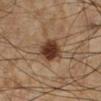Clinical impression:
Imaged during a routine full-body skin examination; the lesion was not biopsied and no histopathology is available.
Background:
Cropped from a total-body skin-imaging series; the visible field is about 15 mm. Captured under cross-polarized illumination. A male subject, roughly 60 years of age. The lesion's longest dimension is about 4 mm. The total-body-photography lesion software estimated an outline eccentricity of about 0.7 (0 = round, 1 = elongated) and a shape-asymmetry score of about 0.2 (0 = symmetric). The analysis additionally found a color-variation rating of about 4/10 and a peripheral color-asymmetry measure near 1. And it measured an automated nevus-likeness rating near 100 out of 100 and a lesion-detection confidence of about 100/100. On the left lower leg.